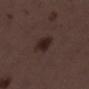biopsy_status: not biopsied; imaged during a skin examination
patient:
  sex: female
  age_approx: 50
lesion_size:
  long_diameter_mm_approx: 2.5
lighting: white-light
image:
  source: total-body photography crop
  field_of_view_mm: 15
site: left lower leg